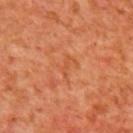Q: What did automated image analysis measure?
A: a mean CIELAB color near L≈54 a*≈31 b*≈42 and roughly 6 lightness units darker than nearby skin; border irregularity of about 4.5 on a 0–10 scale, internal color variation of about 0 on a 0–10 scale, and a peripheral color-asymmetry measure near 0
Q: Who is the patient?
A: female, aged around 40
Q: How was the tile lit?
A: cross-polarized illumination
Q: How was this image acquired?
A: 15 mm crop, total-body photography
Q: Lesion size?
A: ~2.5 mm (longest diameter)
Q: What is the anatomic site?
A: the back